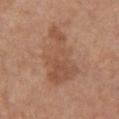Assessment:
This lesion was catalogued during total-body skin photography and was not selected for biopsy.
Background:
Imaged with white-light lighting. The lesion is on the chest. Approximately 7.5 mm at its widest. Automated tile analysis of the lesion measured a lesion area of about 22 mm² and two-axis asymmetry of about 0.4. The software also gave a mean CIELAB color near L≈52 a*≈21 b*≈30 and roughly 8 lightness units darker than nearby skin. The software also gave internal color variation of about 3.5 on a 0–10 scale and a peripheral color-asymmetry measure near 1. A close-up tile cropped from a whole-body skin photograph, about 15 mm across. A female patient in their mid- to late 50s.